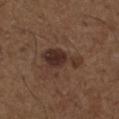<tbp_lesion>
<biopsy_status>not biopsied; imaged during a skin examination</biopsy_status>
</tbp_lesion>